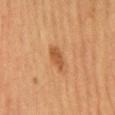The lesion was photographed on a routine skin check and not biopsied; there is no pathology result. About 3 mm across. A female patient, aged 58–62. Automated tile analysis of the lesion measured a within-lesion color-variation index near 2/10 and radial color variation of about 0.5. And it measured an automated nevus-likeness rating near 70 out of 100 and a lesion-detection confidence of about 100/100. On the mid back. Imaged with cross-polarized lighting. Cropped from a total-body skin-imaging series; the visible field is about 15 mm.